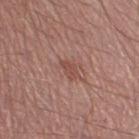No biopsy was performed on this lesion — it was imaged during a full skin examination and was not determined to be concerning. On the right thigh. A 15 mm crop from a total-body photograph taken for skin-cancer surveillance. A male subject roughly 55 years of age.